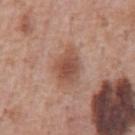No biopsy was performed on this lesion — it was imaged during a full skin examination and was not determined to be concerning.
About 4.5 mm across.
Captured under white-light illumination.
The patient is a male aged approximately 60.
Cropped from a total-body skin-imaging series; the visible field is about 15 mm.
Located on the chest.